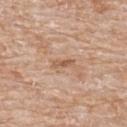Q: What is the imaging modality?
A: ~15 mm tile from a whole-body skin photo
Q: Patient demographics?
A: female, aged approximately 85 or older
Q: Lesion location?
A: the upper back